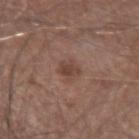Imaged during a routine full-body skin examination; the lesion was not biopsied and no histopathology is available. Measured at roughly 3 mm in maximum diameter. A 15 mm close-up tile from a total-body photography series done for melanoma screening. From the left forearm. Imaged with white-light lighting. A male patient about 50 years old. An algorithmic analysis of the crop reported an area of roughly 4.5 mm² and a shape eccentricity near 0.6. It also reported a border-irregularity index near 2/10, internal color variation of about 2.5 on a 0–10 scale, and peripheral color asymmetry of about 1. It also reported a nevus-likeness score of about 45/100 and a detector confidence of about 100 out of 100 that the crop contains a lesion.